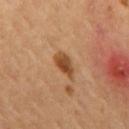  biopsy_status: not biopsied; imaged during a skin examination
  patient:
    sex: male
    age_approx: 55
  automated_metrics:
    cielab_L: 43
    cielab_a: 20
    cielab_b: 34
    vs_skin_darker_L: 11.0
    vs_skin_contrast_norm: 9.0
    border_irregularity_0_10: 2.5
    color_variation_0_10: 3.5
    peripheral_color_asymmetry: 1.0
  image:
    source: total-body photography crop
    field_of_view_mm: 15
  site: mid back